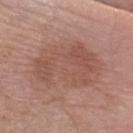Q: Is there a histopathology result?
A: total-body-photography surveillance lesion; no biopsy
Q: What is the imaging modality?
A: 15 mm crop, total-body photography
Q: What are the patient's age and sex?
A: female, aged 73–77
Q: Lesion location?
A: the right forearm